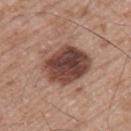notes: no biopsy performed (imaged during a skin exam) | body site: the left upper arm | subject: male, about 65 years old | image source: ~15 mm tile from a whole-body skin photo | diameter: about 6 mm.